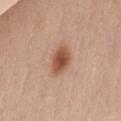<lesion>
<biopsy_status>not biopsied; imaged during a skin examination</biopsy_status>
<site>chest</site>
<patient>
  <sex>female</sex>
  <age_approx>40</age_approx>
</patient>
<lesion_size>
  <long_diameter_mm_approx>4.0</long_diameter_mm_approx>
</lesion_size>
<image>
  <source>total-body photography crop</source>
  <field_of_view_mm>15</field_of_view_mm>
</image>
<automated_metrics>
  <cielab_L>53</cielab_L>
  <cielab_a>23</cielab_a>
  <cielab_b>32</cielab_b>
  <vs_skin_darker_L>14.0</vs_skin_darker_L>
  <vs_skin_contrast_norm>9.5</vs_skin_contrast_norm>
  <border_irregularity_0_10>1.5</border_irregularity_0_10>
  <color_variation_0_10>3.5</color_variation_0_10>
  <peripheral_color_asymmetry>1.0</peripheral_color_asymmetry>
</automated_metrics>
</lesion>